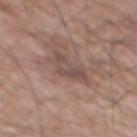Findings:
- workup · catalogued during a skin exam; not biopsied
- subject · male, aged around 55
- imaging modality · 15 mm crop, total-body photography
- body site · the mid back
- lighting · white-light